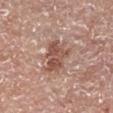{
  "biopsy_status": "not biopsied; imaged during a skin examination",
  "lesion_size": {
    "long_diameter_mm_approx": 4.0
  },
  "site": "left lower leg",
  "lighting": "white-light",
  "image": {
    "source": "total-body photography crop",
    "field_of_view_mm": 15
  },
  "patient": {
    "sex": "male",
    "age_approx": 55
  }
}